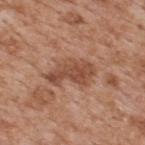Notes:
– biopsy status: total-body-photography surveillance lesion; no biopsy
– location: the upper back
– lighting: white-light
– acquisition: ~15 mm tile from a whole-body skin photo
– patient: male, approximately 65 years of age